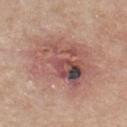Imaged during a routine full-body skin examination; the lesion was not biopsied and no histopathology is available. The patient is a male roughly 55 years of age. On the chest. The recorded lesion diameter is about 9 mm. A 15 mm crop from a total-body photograph taken for skin-cancer surveillance. Captured under white-light illumination.